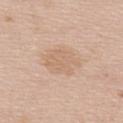The lesion was tiled from a total-body skin photograph and was not biopsied.
On the upper back.
The tile uses white-light illumination.
A roughly 15 mm field-of-view crop from a total-body skin photograph.
Approximately 5 mm at its widest.
A female patient, about 50 years old.